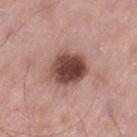Imaged during a routine full-body skin examination; the lesion was not biopsied and no histopathology is available. A male patient aged 53 to 57. Captured under white-light illumination. A close-up tile cropped from a whole-body skin photograph, about 15 mm across. On the left thigh.